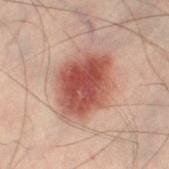  biopsy_status: not biopsied; imaged during a skin examination
  site: left thigh
  patient:
    sex: male
    age_approx: 50
  automated_metrics:
    area_mm2_approx: 24.0
    shape_asymmetry: 0.15
    cielab_L: 42
    cielab_a: 22
    cielab_b: 24
    vs_skin_darker_L: 13.0
    vs_skin_contrast_norm: 10.5
  image:
    source: total-body photography crop
    field_of_view_mm: 15
  lesion_size:
    long_diameter_mm_approx: 7.0